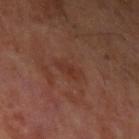follow-up: catalogued during a skin exam; not biopsied | automated metrics: border irregularity of about 3.5 on a 0–10 scale, internal color variation of about 1.5 on a 0–10 scale, and peripheral color asymmetry of about 0.5; an automated nevus-likeness rating near 0 out of 100 and a detector confidence of about 100 out of 100 that the crop contains a lesion | anatomic site: the left upper arm | tile lighting: cross-polarized | patient: male, aged 63 to 67 | image source: 15 mm crop, total-body photography.